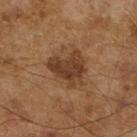workup = no biopsy performed (imaged during a skin exam) | illumination = cross-polarized | subject = male, approximately 65 years of age | imaging modality = ~15 mm tile from a whole-body skin photo.